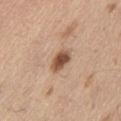notes: total-body-photography surveillance lesion; no biopsy
automated metrics: an area of roughly 5 mm², an eccentricity of roughly 0.8, and a shape-asymmetry score of about 0.25 (0 = symmetric); a lesion color around L≈52 a*≈20 b*≈31 in CIELAB and about 16 CIELAB-L* units darker than the surrounding skin; a classifier nevus-likeness of about 95/100 and lesion-presence confidence of about 100/100
imaging modality: 15 mm crop, total-body photography
illumination: white-light illumination
subject: male, aged around 70
diameter: about 3 mm
location: the abdomen